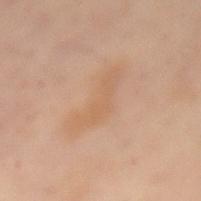• site: the mid back
• automated lesion analysis: an area of roughly 10 mm² and two-axis asymmetry of about 0.45; roughly 5 lightness units darker than nearby skin and a normalized lesion–skin contrast near 4.5; a border-irregularity rating of about 6/10
• tile lighting: cross-polarized
• lesion size: ≈6.5 mm
• image source: ~15 mm crop, total-body skin-cancer survey
• patient: female, in their mid-50s